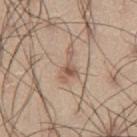size = ~4 mm (longest diameter) | patient = male, approximately 55 years of age | image = 15 mm crop, total-body photography | location = the left thigh.